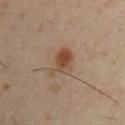The lesion was tiled from a total-body skin photograph and was not biopsied. The lesion is located on the right upper arm. A male patient, aged around 40. Cropped from a total-body skin-imaging series; the visible field is about 15 mm.